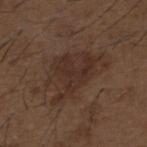Imaged during a routine full-body skin examination; the lesion was not biopsied and no histopathology is available. A male subject, aged around 50. The lesion is on the upper back. A roughly 15 mm field-of-view crop from a total-body skin photograph. Automated tile analysis of the lesion measured a border-irregularity rating of about 5.5/10, a within-lesion color-variation index near 3/10, and a peripheral color-asymmetry measure near 1.